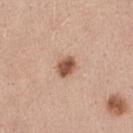A female patient aged 43–47. From the leg. Approximately 2.5 mm at its widest. This is a white-light tile. An algorithmic analysis of the crop reported a lesion area of about 4.5 mm², an outline eccentricity of about 0.65 (0 = round, 1 = elongated), and a symmetry-axis asymmetry near 0.25. It also reported a classifier nevus-likeness of about 95/100. Cropped from a whole-body photographic skin survey; the tile spans about 15 mm.